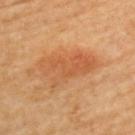Clinical impression:
The lesion was photographed on a routine skin check and not biopsied; there is no pathology result.
Context:
The lesion is on the left upper arm. The subject is a female aged approximately 65. Captured under cross-polarized illumination. Cropped from a total-body skin-imaging series; the visible field is about 15 mm.